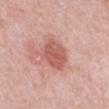Findings:
- workup · no biopsy performed (imaged during a skin exam)
- subject · male, approximately 50 years of age
- location · the chest
- image source · 15 mm crop, total-body photography